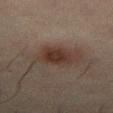<case>
<biopsy_status>not biopsied; imaged during a skin examination</biopsy_status>
<lesion_size>
  <long_diameter_mm_approx>3.0</long_diameter_mm_approx>
</lesion_size>
<site>leg</site>
<patient>
  <sex>male</sex>
  <age_approx>50</age_approx>
</patient>
<image>
  <source>total-body photography crop</source>
  <field_of_view_mm>15</field_of_view_mm>
</image>
<lighting>cross-polarized</lighting>
<automated_metrics>
  <area_mm2_approx>6.5</area_mm2_approx>
  <eccentricity>0.15</eccentricity>
  <shape_asymmetry>0.2</shape_asymmetry>
  <border_irregularity_0_10>2.0</border_irregularity_0_10>
  <peripheral_color_asymmetry>1.0</peripheral_color_asymmetry>
  <lesion_detection_confidence_0_100>100</lesion_detection_confidence_0_100>
</automated_metrics>
</case>